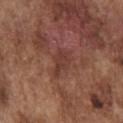Recorded during total-body skin imaging; not selected for excision or biopsy. A male subject, roughly 75 years of age. On the chest. Cropped from a total-body skin-imaging series; the visible field is about 15 mm. Captured under white-light illumination.